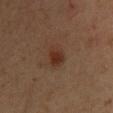Imaged during a routine full-body skin examination; the lesion was not biopsied and no histopathology is available. The total-body-photography lesion software estimated a shape eccentricity near 0.4 and two-axis asymmetry of about 0.25. It also reported an automated nevus-likeness rating near 90 out of 100 and lesion-presence confidence of about 100/100. A 15 mm close-up tile from a total-body photography series done for melanoma screening. Longest diameter approximately 2.5 mm. A male subject approximately 35 years of age. Located on the chest. This is a cross-polarized tile.